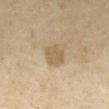This lesion was catalogued during total-body skin photography and was not selected for biopsy. On the mid back. Measured at roughly 3 mm in maximum diameter. Cropped from a total-body skin-imaging series; the visible field is about 15 mm. The lesion-visualizer software estimated border irregularity of about 2 on a 0–10 scale, internal color variation of about 1.5 on a 0–10 scale, and peripheral color asymmetry of about 0.5. The software also gave an automated nevus-likeness rating near 5 out of 100. The tile uses cross-polarized illumination. The subject is a female aged approximately 60.